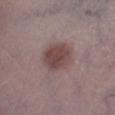- notes — no biopsy performed (imaged during a skin exam)
- subject — male, roughly 55 years of age
- site — the right lower leg
- acquisition — 15 mm crop, total-body photography